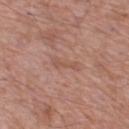Assessment:
The lesion was tiled from a total-body skin photograph and was not biopsied.
Clinical summary:
Located on the left lower leg. A male patient, about 55 years old. A close-up tile cropped from a whole-body skin photograph, about 15 mm across.